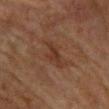{
  "image": {
    "source": "total-body photography crop",
    "field_of_view_mm": 15
  },
  "site": "front of the torso",
  "patient": {
    "sex": "female",
    "age_approx": 80
  },
  "lesion_size": {
    "long_diameter_mm_approx": 4.0
  }
}